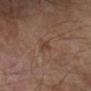workup: catalogued during a skin exam; not biopsied | lighting: cross-polarized illumination | TBP lesion metrics: a lesion area of about 1 mm², a shape eccentricity near 0.85, and a shape-asymmetry score of about 0.45 (0 = symmetric); a border-irregularity index near 3.5/10, a within-lesion color-variation index near 0/10, and peripheral color asymmetry of about 0; lesion-presence confidence of about 100/100 | anatomic site: the arm | diameter: ~1.5 mm (longest diameter) | patient: male, roughly 65 years of age | image: ~15 mm tile from a whole-body skin photo.